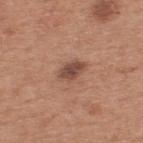{"biopsy_status": "not biopsied; imaged during a skin examination", "patient": {"sex": "male", "age_approx": 55}, "image": {"source": "total-body photography crop", "field_of_view_mm": 15}, "automated_metrics": {"border_irregularity_0_10": 2.0, "color_variation_0_10": 3.0, "peripheral_color_asymmetry": 1.0, "nevus_likeness_0_100": 65, "lesion_detection_confidence_0_100": 100}, "site": "upper back", "lesion_size": {"long_diameter_mm_approx": 3.5}}